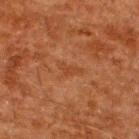notes: no biopsy performed (imaged during a skin exam)
patient: male, aged around 60
diameter: about 3 mm
imaging modality: ~15 mm crop, total-body skin-cancer survey
body site: the upper back